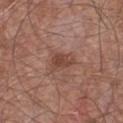workup — imaged on a skin check; not biopsied
size — about 3.5 mm
subject — male, in their mid- to late 60s
anatomic site — the front of the torso
lighting — white-light
acquisition — ~15 mm tile from a whole-body skin photo
automated lesion analysis — an area of roughly 6 mm² and an eccentricity of roughly 0.75; border irregularity of about 3.5 on a 0–10 scale and radial color variation of about 1